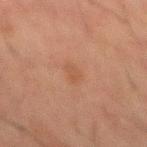Impression: Part of a total-body skin-imaging series; this lesion was reviewed on a skin check and was not flagged for biopsy. Clinical summary: This image is a 15 mm lesion crop taken from a total-body photograph. The lesion is located on the mid back. A male patient, about 50 years old.